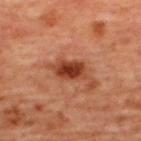<lesion>
<biopsy_status>not biopsied; imaged during a skin examination</biopsy_status>
</lesion>